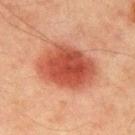Recorded during total-body skin imaging; not selected for excision or biopsy. Imaged with cross-polarized lighting. A close-up tile cropped from a whole-body skin photograph, about 15 mm across. The lesion is on the chest. The recorded lesion diameter is about 7 mm. The subject is a male about 65 years old.